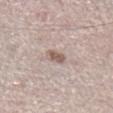workup=no biopsy performed (imaged during a skin exam)
size=about 2.5 mm
site=the right lower leg
tile lighting=white-light illumination
imaging modality=15 mm crop, total-body photography
subject=male, in their 60s